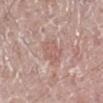Part of a total-body skin-imaging series; this lesion was reviewed on a skin check and was not flagged for biopsy. On the right leg. Cropped from a whole-body photographic skin survey; the tile spans about 15 mm. A male patient about 70 years old.